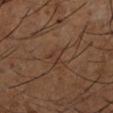Assessment:
The lesion was photographed on a routine skin check and not biopsied; there is no pathology result.
Context:
The lesion is on the left lower leg. The tile uses cross-polarized illumination. Cropped from a whole-body photographic skin survey; the tile spans about 15 mm. A male patient about 65 years old. The lesion-visualizer software estimated a lesion area of about 3.5 mm² and an outline eccentricity of about 0.8 (0 = round, 1 = elongated). The software also gave a mean CIELAB color near L≈35 a*≈17 b*≈26, a lesion–skin lightness drop of about 5, and a normalized border contrast of about 4.5. And it measured border irregularity of about 3.5 on a 0–10 scale, internal color variation of about 1.5 on a 0–10 scale, and radial color variation of about 0.5.